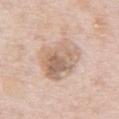biopsy_status: not biopsied; imaged during a skin examination
lesion_size:
  long_diameter_mm_approx: 6.5
image:
  source: total-body photography crop
  field_of_view_mm: 15
patient:
  sex: male
  age_approx: 75
lighting: white-light
site: abdomen